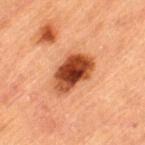notes=imaged on a skin check; not biopsied
body site=the leg
subject=male, aged approximately 85 or older
imaging modality=~15 mm tile from a whole-body skin photo
diameter=~5.5 mm (longest diameter)